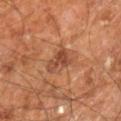follow-up = catalogued during a skin exam; not biopsied | subject = male, approximately 60 years of age | site = the right leg | image = total-body-photography crop, ~15 mm field of view.